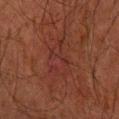{"biopsy_status": "not biopsied; imaged during a skin examination", "image": {"source": "total-body photography crop", "field_of_view_mm": 15}, "automated_metrics": {"border_irregularity_0_10": 7.5, "color_variation_0_10": 4.0, "peripheral_color_asymmetry": 1.5, "nevus_likeness_0_100": 0}, "site": "left forearm", "lesion_size": {"long_diameter_mm_approx": 8.0}, "patient": {"sex": "male", "age_approx": 65}}